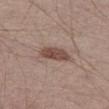Case summary:
* follow-up — no biopsy performed (imaged during a skin exam)
* image source — ~15 mm tile from a whole-body skin photo
* subject — male, in their mid-50s
* body site — the leg
* illumination — white-light